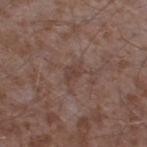Imaged during a routine full-body skin examination; the lesion was not biopsied and no histopathology is available.
From the right thigh.
An algorithmic analysis of the crop reported lesion-presence confidence of about 100/100.
A male subject about 45 years old.
A 15 mm close-up extracted from a 3D total-body photography capture.
Longest diameter approximately 2.5 mm.
The tile uses white-light illumination.